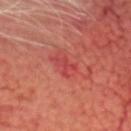biopsy_status: not biopsied; imaged during a skin examination